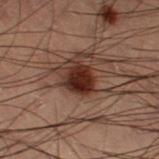follow-up: total-body-photography surveillance lesion; no biopsy | subject: male, roughly 55 years of age | image-analysis metrics: a classifier nevus-likeness of about 95/100 and a detector confidence of about 100 out of 100 that the crop contains a lesion | size: about 3.5 mm | site: the right thigh | image source: ~15 mm tile from a whole-body skin photo | tile lighting: cross-polarized.